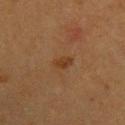notes = catalogued during a skin exam; not biopsied | acquisition = ~15 mm tile from a whole-body skin photo | patient = female, aged around 40 | location = the upper back.